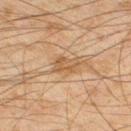Assessment:
Recorded during total-body skin imaging; not selected for excision or biopsy.
Image and clinical context:
The lesion is located on the left thigh. A roughly 15 mm field-of-view crop from a total-body skin photograph. A male subject aged around 45.